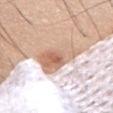The lesion was photographed on a routine skin check and not biopsied; there is no pathology result. About 6 mm across. A male subject, approximately 60 years of age. Cropped from a total-body skin-imaging series; the visible field is about 15 mm. The lesion is located on the abdomen.